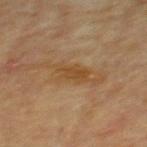Captured during whole-body skin photography for melanoma surveillance; the lesion was not biopsied.
From the mid back.
A roughly 15 mm field-of-view crop from a total-body skin photograph.
A male subject, approximately 65 years of age.
The lesion-visualizer software estimated a footprint of about 5 mm², an outline eccentricity of about 0.9 (0 = round, 1 = elongated), and two-axis asymmetry of about 0.3. The analysis additionally found a lesion color around L≈41 a*≈17 b*≈34 in CIELAB and roughly 7 lightness units darker than nearby skin. The software also gave a border-irregularity index near 3.5/10 and a within-lesion color-variation index near 2/10.
Imaged with cross-polarized lighting.
Measured at roughly 4 mm in maximum diameter.